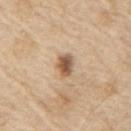Findings:
* biopsy status · imaged on a skin check; not biopsied
* acquisition · 15 mm crop, total-body photography
* tile lighting · white-light illumination
* TBP lesion metrics · a footprint of about 4.5 mm², an eccentricity of roughly 0.8, and a symmetry-axis asymmetry near 0.3; a lesion color around L≈56 a*≈17 b*≈33 in CIELAB, about 16 CIELAB-L* units darker than the surrounding skin, and a normalized lesion–skin contrast near 10; a color-variation rating of about 5/10 and radial color variation of about 1.5; an automated nevus-likeness rating near 95 out of 100 and a lesion-detection confidence of about 100/100
* subject · male, aged around 70
* anatomic site · the arm